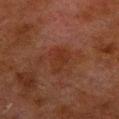- notes — catalogued during a skin exam; not biopsied
- tile lighting — cross-polarized
- automated lesion analysis — a lesion area of about 7 mm² and an eccentricity of roughly 0.75; a lesion color around L≈25 a*≈20 b*≈24 in CIELAB, about 4 CIELAB-L* units darker than the surrounding skin, and a normalized lesion–skin contrast near 5.5
- imaging modality — ~15 mm tile from a whole-body skin photo
- patient — male, approximately 80 years of age
- site — the right lower leg
- diameter — ≈3.5 mm